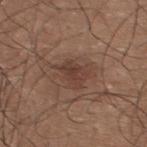Part of a total-body skin-imaging series; this lesion was reviewed on a skin check and was not flagged for biopsy.
On the upper back.
This image is a 15 mm lesion crop taken from a total-body photograph.
Longest diameter approximately 3.5 mm.
The patient is a male roughly 25 years of age.